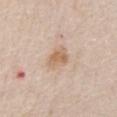{
  "biopsy_status": "not biopsied; imaged during a skin examination"
}